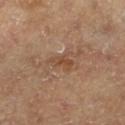<record>
<biopsy_status>not biopsied; imaged during a skin examination</biopsy_status>
<patient>
  <sex>male</sex>
  <age_approx>65</age_approx>
</patient>
<image>
  <source>total-body photography crop</source>
  <field_of_view_mm>15</field_of_view_mm>
</image>
<automated_metrics>
  <border_irregularity_0_10>4.0</border_irregularity_0_10>
  <color_variation_0_10>2.0</color_variation_0_10>
  <peripheral_color_asymmetry>0.5</peripheral_color_asymmetry>
  <nevus_likeness_0_100>0</nevus_likeness_0_100>
  <lesion_detection_confidence_0_100>100</lesion_detection_confidence_0_100>
</automated_metrics>
<site>right lower leg</site>
<lesion_size>
  <long_diameter_mm_approx>3.0</long_diameter_mm_approx>
</lesion_size>
</record>